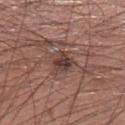The lesion was tiled from a total-body skin photograph and was not biopsied.
The total-body-photography lesion software estimated an area of roughly 4 mm² and an outline eccentricity of about 0.65 (0 = round, 1 = elongated). It also reported an average lesion color of about L≈38 a*≈19 b*≈21 (CIELAB), a lesion–skin lightness drop of about 11, and a normalized lesion–skin contrast near 9.5. It also reported an automated nevus-likeness rating near 0 out of 100 and a lesion-detection confidence of about 100/100.
Cropped from a total-body skin-imaging series; the visible field is about 15 mm.
Located on the leg.
A male patient, in their mid- to late 40s.
This is a white-light tile.